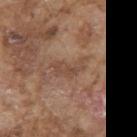Assessment:
The lesion was tiled from a total-body skin photograph and was not biopsied.
Clinical summary:
This is a white-light tile. The subject is a male roughly 75 years of age. The recorded lesion diameter is about 4 mm. Cropped from a total-body skin-imaging series; the visible field is about 15 mm. From the arm.